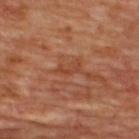{"lighting": "cross-polarized", "site": "upper back", "image": {"source": "total-body photography crop", "field_of_view_mm": 15}, "patient": {"sex": "female", "age_approx": 45}}